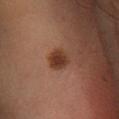imaging modality — 15 mm crop, total-body photography; body site — the left lower leg; patient — male, roughly 60 years of age.